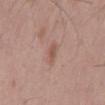<record>
<biopsy_status>not biopsied; imaged during a skin examination</biopsy_status>
<site>mid back</site>
<image>
  <source>total-body photography crop</source>
  <field_of_view_mm>15</field_of_view_mm>
</image>
<patient>
  <sex>male</sex>
  <age_approx>55</age_approx>
</patient>
<automated_metrics>
  <cielab_L>54</cielab_L>
  <cielab_a>20</cielab_a>
  <cielab_b>27</cielab_b>
  <vs_skin_darker_L>8.0</vs_skin_darker_L>
  <vs_skin_contrast_norm>6.0</vs_skin_contrast_norm>
  <border_irregularity_0_10>3.0</border_irregularity_0_10>
  <peripheral_color_asymmetry>0.5</peripheral_color_asymmetry>
  <nevus_likeness_0_100>0</nevus_likeness_0_100>
  <lesion_detection_confidence_0_100>100</lesion_detection_confidence_0_100>
</automated_metrics>
<lesion_size>
  <long_diameter_mm_approx>2.5</long_diameter_mm_approx>
</lesion_size>
</record>